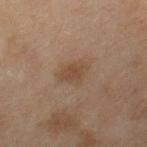Assessment: Part of a total-body skin-imaging series; this lesion was reviewed on a skin check and was not flagged for biopsy. Acquisition and patient details: The lesion is located on the right thigh. The recorded lesion diameter is about 3.5 mm. Automated tile analysis of the lesion measured a classifier nevus-likeness of about 40/100 and a detector confidence of about 100 out of 100 that the crop contains a lesion. A female subject approximately 60 years of age. A lesion tile, about 15 mm wide, cut from a 3D total-body photograph.